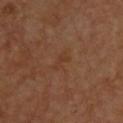Imaged during a routine full-body skin examination; the lesion was not biopsied and no histopathology is available. A male patient aged 58–62. The recorded lesion diameter is about 2.5 mm. Captured under cross-polarized illumination. The lesion is on the upper back. A 15 mm close-up tile from a total-body photography series done for melanoma screening.